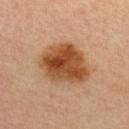A female subject, in their mid- to late 40s.
The lesion is located on the upper back.
Longest diameter approximately 6 mm.
An algorithmic analysis of the crop reported a classifier nevus-likeness of about 100/100.
A 15 mm crop from a total-body photograph taken for skin-cancer surveillance.
This is a cross-polarized tile.